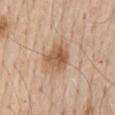Captured during whole-body skin photography for melanoma surveillance; the lesion was not biopsied. The lesion is located on the mid back. The total-body-photography lesion software estimated a lesion area of about 9 mm², a shape eccentricity near 0.4, and a symmetry-axis asymmetry near 0.25. It also reported a lesion color around L≈57 a*≈19 b*≈32 in CIELAB, about 11 CIELAB-L* units darker than the surrounding skin, and a normalized border contrast of about 7.5. And it measured a lesion-detection confidence of about 100/100. The lesion's longest dimension is about 3.5 mm. The patient is a male approximately 60 years of age. A 15 mm crop from a total-body photograph taken for skin-cancer surveillance. Captured under white-light illumination.